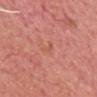This lesion was catalogued during total-body skin photography and was not selected for biopsy. The subject is a male aged approximately 75. Located on the head or neck. A 15 mm close-up extracted from a 3D total-body photography capture.